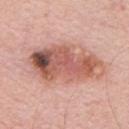{"biopsy_status": "not biopsied; imaged during a skin examination", "patient": {"sex": "male", "age_approx": 65}, "lesion_size": {"long_diameter_mm_approx": 9.5}, "image": {"source": "total-body photography crop", "field_of_view_mm": 15}, "site": "chest"}